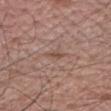notes: no biopsy performed (imaged during a skin exam) | illumination: white-light | image: total-body-photography crop, ~15 mm field of view | subject: male, aged approximately 65 | anatomic site: the right upper arm.